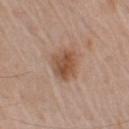Impression:
No biopsy was performed on this lesion — it was imaged during a full skin examination and was not determined to be concerning.
Context:
On the left upper arm. Imaged with white-light lighting. A male patient aged approximately 65. Approximately 4 mm at its widest. A 15 mm crop from a total-body photograph taken for skin-cancer surveillance.